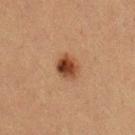biopsy_status: not biopsied; imaged during a skin examination
lighting: cross-polarized
site: left upper arm
patient:
  sex: male
  age_approx: 50
lesion_size:
  long_diameter_mm_approx: 3.0
image:
  source: total-body photography crop
  field_of_view_mm: 15
automated_metrics:
  area_mm2_approx: 6.0
  eccentricity: 0.55
  shape_asymmetry: 0.2